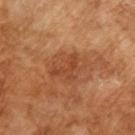Part of a total-body skin-imaging series; this lesion was reviewed on a skin check and was not flagged for biopsy. The tile uses cross-polarized illumination. A 15 mm close-up extracted from a 3D total-body photography capture. The total-body-photography lesion software estimated a symmetry-axis asymmetry near 0.25. And it measured a border-irregularity index near 3.5/10, a within-lesion color-variation index near 3.5/10, and radial color variation of about 1.5. A male subject, about 65 years old.